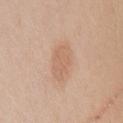* size · ≈4 mm
* patient · female, aged 38–42
* body site · the front of the torso
* acquisition · total-body-photography crop, ~15 mm field of view